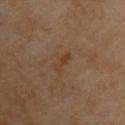subject: male, in their mid- to late 50s | anatomic site: the upper back | lesion size: ≈3 mm | image-analysis metrics: a mean CIELAB color near L≈40 a*≈17 b*≈31, about 6 CIELAB-L* units darker than the surrounding skin, and a normalized lesion–skin contrast near 6; a border-irregularity index near 3.5/10, a color-variation rating of about 0/10, and radial color variation of about 0; a lesion-detection confidence of about 100/100 | image source: ~15 mm tile from a whole-body skin photo | lighting: cross-polarized illumination.